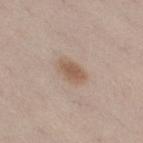biopsy_status: not biopsied; imaged during a skin examination
lesion_size:
  long_diameter_mm_approx: 3.5
automated_metrics:
  cielab_L: 57
  cielab_a: 16
  cielab_b: 28
  vs_skin_darker_L: 9.0
  vs_skin_contrast_norm: 7.0
  border_irregularity_0_10: 2.0
  color_variation_0_10: 2.5
  peripheral_color_asymmetry: 1.0
  nevus_likeness_0_100: 90
  lesion_detection_confidence_0_100: 100
lighting: white-light
image:
  source: total-body photography crop
  field_of_view_mm: 15
patient:
  sex: female
  age_approx: 40
site: right thigh